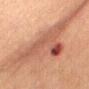Assessment: The lesion was tiled from a total-body skin photograph and was not biopsied. Context: A female subject about 60 years old. From the chest. A 15 mm crop from a total-body photograph taken for skin-cancer surveillance.